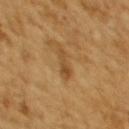Captured during whole-body skin photography for melanoma surveillance; the lesion was not biopsied.
A female subject, aged 53–57.
Imaged with cross-polarized lighting.
Longest diameter approximately 3 mm.
Cropped from a total-body skin-imaging series; the visible field is about 15 mm.
The lesion is on the back.